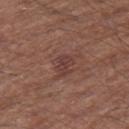workup: catalogued during a skin exam; not biopsied | lesion size: about 2.5 mm | acquisition: 15 mm crop, total-body photography | body site: the right thigh | patient: male, aged approximately 65 | image-analysis metrics: an area of roughly 3.5 mm² and two-axis asymmetry of about 0.4; a mean CIELAB color near L≈38 a*≈21 b*≈22, a lesion–skin lightness drop of about 7, and a lesion-to-skin contrast of about 6.5 (normalized; higher = more distinct); a border-irregularity rating of about 3.5/10, a within-lesion color-variation index near 1/10, and a peripheral color-asymmetry measure near 0.5 | lighting: white-light illumination.